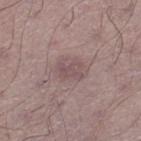Part of a total-body skin-imaging series; this lesion was reviewed on a skin check and was not flagged for biopsy.
An algorithmic analysis of the crop reported a lesion color around L≈50 a*≈17 b*≈16 in CIELAB and a normalized border contrast of about 5.5. And it measured an automated nevus-likeness rating near 0 out of 100 and lesion-presence confidence of about 95/100.
The tile uses white-light illumination.
The patient is a male aged 58 to 62.
Located on the right lower leg.
This image is a 15 mm lesion crop taken from a total-body photograph.